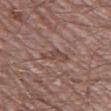Imaged during a routine full-body skin examination; the lesion was not biopsied and no histopathology is available. The patient is a male aged 63 to 67. A lesion tile, about 15 mm wide, cut from a 3D total-body photograph. Approximately 3 mm at its widest. Imaged with white-light lighting. Located on the left thigh.